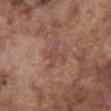Impression:
Part of a total-body skin-imaging series; this lesion was reviewed on a skin check and was not flagged for biopsy.
Context:
The total-body-photography lesion software estimated a footprint of about 5 mm² and a shape eccentricity near 0.55. And it measured a color-variation rating of about 1.5/10 and peripheral color asymmetry of about 0.5. The analysis additionally found a classifier nevus-likeness of about 0/100 and a detector confidence of about 100 out of 100 that the crop contains a lesion. A male patient, aged 73–77. Measured at roughly 3 mm in maximum diameter. A 15 mm close-up extracted from a 3D total-body photography capture. The tile uses white-light illumination. On the abdomen.